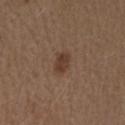follow-up — no biopsy performed (imaged during a skin exam).